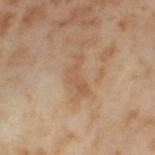No biopsy was performed on this lesion — it was imaged during a full skin examination and was not determined to be concerning. The recorded lesion diameter is about 4 mm. Automated image analysis of the tile measured a footprint of about 5 mm², a shape eccentricity near 0.9, and a shape-asymmetry score of about 0.7 (0 = symmetric). The software also gave an average lesion color of about L≈56 a*≈19 b*≈33 (CIELAB), about 7 CIELAB-L* units darker than the surrounding skin, and a lesion-to-skin contrast of about 5.5 (normalized; higher = more distinct). It also reported a classifier nevus-likeness of about 0/100 and lesion-presence confidence of about 100/100. Located on the left thigh. A 15 mm close-up tile from a total-body photography series done for melanoma screening. A female patient aged approximately 55. The tile uses cross-polarized illumination.